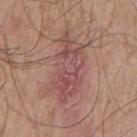Findings:
– follow-up: total-body-photography surveillance lesion; no biopsy
– body site: the chest
– patient: male, aged around 55
– size: ~8.5 mm (longest diameter)
– tile lighting: white-light
– TBP lesion metrics: an area of roughly 22 mm², an eccentricity of roughly 0.9, and two-axis asymmetry of about 0.35
– acquisition: ~15 mm crop, total-body skin-cancer survey